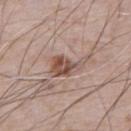Case summary:
• notes · no biopsy performed (imaged during a skin exam)
• subject · male, aged 78–82
• tile lighting · white-light illumination
• size · about 4 mm
• site · the front of the torso
• image · ~15 mm crop, total-body skin-cancer survey